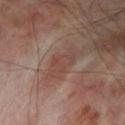Recorded during total-body skin imaging; not selected for excision or biopsy. Captured under cross-polarized illumination. Automated tile analysis of the lesion measured a lesion–skin lightness drop of about 6 and a normalized lesion–skin contrast near 5. The software also gave a classifier nevus-likeness of about 0/100. Located on the left thigh. A lesion tile, about 15 mm wide, cut from a 3D total-body photograph. A male patient approximately 70 years of age.